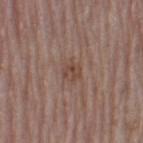notes = no biopsy performed (imaged during a skin exam) | location = the left thigh | image source = 15 mm crop, total-body photography | patient = female, in their mid-60s.